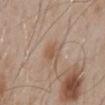| feature | finding |
|---|---|
| biopsy status | catalogued during a skin exam; not biopsied |
| patient | male, in their mid-50s |
| image-analysis metrics | a lesion color around L≈55 a*≈17 b*≈29 in CIELAB and roughly 7 lightness units darker than nearby skin; an automated nevus-likeness rating near 0 out of 100 |
| site | the back |
| acquisition | total-body-photography crop, ~15 mm field of view |
| illumination | white-light |